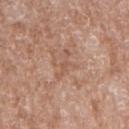Findings:
• notes: total-body-photography surveillance lesion; no biopsy
• imaging modality: 15 mm crop, total-body photography
• illumination: white-light
• lesion size: about 4 mm
• body site: the right upper arm
• patient: female, aged around 75
• automated metrics: a lesion area of about 4 mm², an eccentricity of roughly 0.9, and a shape-asymmetry score of about 0.65 (0 = symmetric); a nevus-likeness score of about 0/100